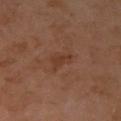tile lighting=cross-polarized; automated lesion analysis=an automated nevus-likeness rating near 0 out of 100; acquisition=~15 mm crop, total-body skin-cancer survey; size=≈3 mm; subject=female, in their mid-50s; site=the right upper arm.